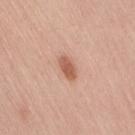Clinical summary: About 3 mm across. Automated image analysis of the tile measured a footprint of about 4 mm², an eccentricity of roughly 0.85, and a symmetry-axis asymmetry near 0.2. The analysis additionally found an average lesion color of about L≈58 a*≈24 b*≈31 (CIELAB), a lesion–skin lightness drop of about 12, and a normalized lesion–skin contrast near 8. It also reported a border-irregularity index near 1.5/10, internal color variation of about 2 on a 0–10 scale, and radial color variation of about 0.5. The analysis additionally found lesion-presence confidence of about 100/100. The subject is a female aged approximately 50. The tile uses white-light illumination. Cropped from a whole-body photographic skin survey; the tile spans about 15 mm. Located on the right thigh.